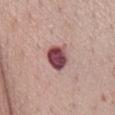Part of a total-body skin-imaging series; this lesion was reviewed on a skin check and was not flagged for biopsy. A roughly 15 mm field-of-view crop from a total-body skin photograph. Captured under white-light illumination. A male patient aged 68 to 72. The lesion is located on the chest. About 4 mm across.